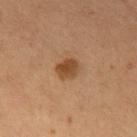follow-up: total-body-photography surveillance lesion; no biopsy | diameter: ≈2.5 mm | imaging modality: total-body-photography crop, ~15 mm field of view | automated metrics: an outline eccentricity of about 0.4 (0 = round, 1 = elongated) and two-axis asymmetry of about 0.3; a nevus-likeness score of about 95/100 | body site: the abdomen | tile lighting: cross-polarized illumination | subject: male, roughly 55 years of age.